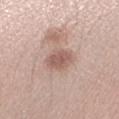workup: imaged on a skin check; not biopsied | subject: female, in their mid-30s | lesion size: about 3 mm | location: the right forearm | illumination: white-light | imaging modality: ~15 mm tile from a whole-body skin photo | image-analysis metrics: an area of roughly 6 mm², a shape eccentricity near 0.6, and two-axis asymmetry of about 0.2; a lesion color around L≈57 a*≈20 b*≈25 in CIELAB and roughly 10 lightness units darker than nearby skin; a border-irregularity index near 2/10 and internal color variation of about 2 on a 0–10 scale; a nevus-likeness score of about 60/100 and lesion-presence confidence of about 100/100.